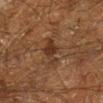No biopsy was performed on this lesion — it was imaged during a full skin examination and was not determined to be concerning. From the left lower leg. A lesion tile, about 15 mm wide, cut from a 3D total-body photograph. The patient is a male roughly 60 years of age.